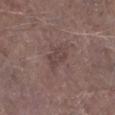notes: catalogued during a skin exam; not biopsied
lighting: white-light illumination
anatomic site: the leg
patient: male, approximately 75 years of age
imaging modality: ~15 mm crop, total-body skin-cancer survey
lesion diameter: about 3 mm
automated metrics: an area of roughly 4.5 mm², an eccentricity of roughly 0.75, and a shape-asymmetry score of about 0.3 (0 = symmetric)